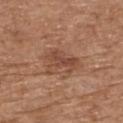biopsy status = total-body-photography surveillance lesion; no biopsy | patient = male, aged around 70 | lesion size = ~4 mm (longest diameter) | body site = the upper back | imaging modality = total-body-photography crop, ~15 mm field of view.